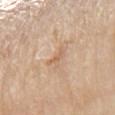No biopsy was performed on this lesion — it was imaged during a full skin examination and was not determined to be concerning.
From the left arm.
A roughly 15 mm field-of-view crop from a total-body skin photograph.
A female patient roughly 65 years of age.
Captured under white-light illumination.
The lesion's longest dimension is about 2.5 mm.
Automated image analysis of the tile measured a lesion color around L≈63 a*≈18 b*≈33 in CIELAB, about 7 CIELAB-L* units darker than the surrounding skin, and a normalized border contrast of about 5. And it measured a border-irregularity rating of about 5.5/10, a color-variation rating of about 0/10, and a peripheral color-asymmetry measure near 0. The analysis additionally found a detector confidence of about 100 out of 100 that the crop contains a lesion.